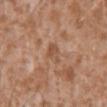follow-up = total-body-photography surveillance lesion; no biopsy
lighting = white-light
automated metrics = an area of roughly 5 mm²; a nevus-likeness score of about 0/100 and lesion-presence confidence of about 100/100
subject = male, aged around 45
image = total-body-photography crop, ~15 mm field of view
anatomic site = the front of the torso